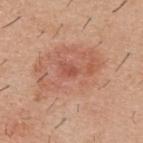<case>
  <biopsy_status>not biopsied; imaged during a skin examination</biopsy_status>
  <image>
    <source>total-body photography crop</source>
    <field_of_view_mm>15</field_of_view_mm>
  </image>
  <lighting>white-light</lighting>
  <site>upper back</site>
  <patient>
    <sex>male</sex>
    <age_approx>40</age_approx>
  </patient>
</case>